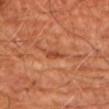Impression: Imaged during a routine full-body skin examination; the lesion was not biopsied and no histopathology is available. Context: A region of skin cropped from a whole-body photographic capture, roughly 15 mm wide. The tile uses cross-polarized illumination. Automated tile analysis of the lesion measured an average lesion color of about L≈45 a*≈29 b*≈35 (CIELAB), roughly 9 lightness units darker than nearby skin, and a normalized border contrast of about 6.5. The analysis additionally found a border-irregularity rating of about 3/10 and a peripheral color-asymmetry measure near 0.5. The lesion's longest dimension is about 2.5 mm. A male patient, approximately 65 years of age. The lesion is located on the arm.